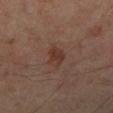Notes:
– automated lesion analysis · a footprint of about 5 mm², an outline eccentricity of about 0.7 (0 = round, 1 = elongated), and two-axis asymmetry of about 0.3; a border-irregularity rating of about 3/10, internal color variation of about 2.5 on a 0–10 scale, and radial color variation of about 1
– patient · male, aged 48–52
– lesion diameter · ~2.5 mm (longest diameter)
– site · the left leg
– image source · ~15 mm crop, total-body skin-cancer survey
– illumination · cross-polarized